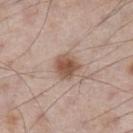A male subject, aged around 60.
Located on the left lower leg.
The tile uses white-light illumination.
Approximately 3 mm at its widest.
An algorithmic analysis of the crop reported a footprint of about 7.5 mm², a shape eccentricity near 0.2, and two-axis asymmetry of about 0.15. And it measured a mean CIELAB color near L≈54 a*≈18 b*≈27 and a lesion–skin lightness drop of about 12.
A close-up tile cropped from a whole-body skin photograph, about 15 mm across.